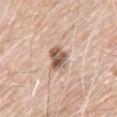Captured during whole-body skin photography for melanoma surveillance; the lesion was not biopsied. A male patient, about 70 years old. From the upper back. A close-up tile cropped from a whole-body skin photograph, about 15 mm across. Imaged with white-light lighting.